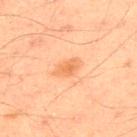workup: total-body-photography surveillance lesion; no biopsy | patient: male, aged 43–47 | acquisition: ~15 mm crop, total-body skin-cancer survey | illumination: cross-polarized | body site: the upper back | size: ~2.5 mm (longest diameter).